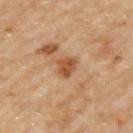The lesion was photographed on a routine skin check and not biopsied; there is no pathology result.